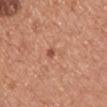biopsy status = total-body-photography surveillance lesion; no biopsy
diameter = ≈3 mm
lighting = white-light illumination
image-analysis metrics = an automated nevus-likeness rating near 0 out of 100 and a lesion-detection confidence of about 100/100
location = the upper back
image source = total-body-photography crop, ~15 mm field of view
patient = female, about 35 years old